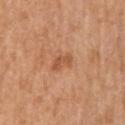Findings:
- notes: total-body-photography surveillance lesion; no biopsy
- acquisition: 15 mm crop, total-body photography
- site: the arm
- patient: male, roughly 70 years of age
- lesion size: ≈3 mm
- automated metrics: a lesion area of about 3.5 mm², an eccentricity of roughly 0.9, and a shape-asymmetry score of about 0.35 (0 = symmetric); internal color variation of about 1.5 on a 0–10 scale and a peripheral color-asymmetry measure near 0.5
- illumination: white-light illumination